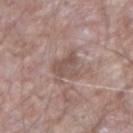<lesion>
  <biopsy_status>not biopsied; imaged during a skin examination</biopsy_status>
  <image>
    <source>total-body photography crop</source>
    <field_of_view_mm>15</field_of_view_mm>
  </image>
  <lighting>white-light</lighting>
  <automated_metrics>
    <cielab_L>51</cielab_L>
    <cielab_a>17</cielab_a>
    <cielab_b>21</cielab_b>
    <vs_skin_darker_L>9.0</vs_skin_darker_L>
    <vs_skin_contrast_norm>6.0</vs_skin_contrast_norm>
    <color_variation_0_10>3.5</color_variation_0_10>
    <peripheral_color_asymmetry>1.0</peripheral_color_asymmetry>
    <nevus_likeness_0_100>0</nevus_likeness_0_100>
    <lesion_detection_confidence_0_100>95</lesion_detection_confidence_0_100>
  </automated_metrics>
  <patient>
    <sex>male</sex>
    <age_approx>65</age_approx>
  </patient>
  <lesion_size>
    <long_diameter_mm_approx>3.5</long_diameter_mm_approx>
  </lesion_size>
  <site>arm</site>
</lesion>